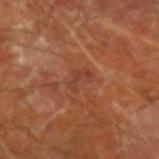Case summary:
* workup — no biopsy performed (imaged during a skin exam)
* image — 15 mm crop, total-body photography
* patient — male, about 65 years old
* site — the arm
* lesion diameter — ~2.5 mm (longest diameter)
* lighting — cross-polarized illumination